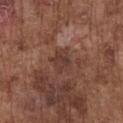Recorded during total-body skin imaging; not selected for excision or biopsy.
Measured at roughly 3 mm in maximum diameter.
A male patient, approximately 75 years of age.
Located on the chest.
A lesion tile, about 15 mm wide, cut from a 3D total-body photograph.